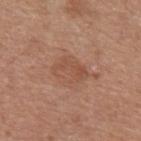Notes:
- image · ~15 mm tile from a whole-body skin photo
- subject · female, aged 48–52
- illumination · white-light illumination
- site · the left upper arm
- diameter · about 4 mm